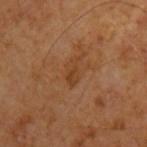{"biopsy_status": "not biopsied; imaged during a skin examination", "lesion_size": {"long_diameter_mm_approx": 3.5}, "site": "left upper arm", "patient": {"sex": "male", "age_approx": 65}, "image": {"source": "total-body photography crop", "field_of_view_mm": 15}, "automated_metrics": {"area_mm2_approx": 3.5, "eccentricity": 0.9, "cielab_L": 38, "cielab_a": 21, "cielab_b": 34, "vs_skin_darker_L": 6.0, "lesion_detection_confidence_0_100": 100}, "lighting": "cross-polarized"}